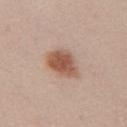The lesion was photographed on a routine skin check and not biopsied; there is no pathology result. A 15 mm crop from a total-body photograph taken for skin-cancer surveillance. A female subject, in their mid-40s. From the chest.